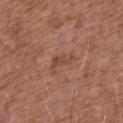• follow-up · total-body-photography surveillance lesion; no biopsy
• patient · male, roughly 75 years of age
• site · the front of the torso
• tile lighting · white-light
• imaging modality · ~15 mm tile from a whole-body skin photo